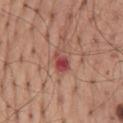biopsy status = imaged on a skin check; not biopsied
acquisition = 15 mm crop, total-body photography
patient = male, approximately 60 years of age
automated lesion analysis = an area of roughly 4.5 mm² and two-axis asymmetry of about 0.3; roughly 11 lightness units darker than nearby skin and a normalized lesion–skin contrast near 8.5; a border-irregularity index near 3/10, internal color variation of about 3.5 on a 0–10 scale, and peripheral color asymmetry of about 1; a classifier nevus-likeness of about 0/100
lesion diameter = about 3 mm
site = the mid back